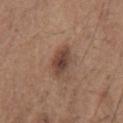Q: Was this lesion biopsied?
A: catalogued during a skin exam; not biopsied
Q: How was the tile lit?
A: white-light
Q: Where on the body is the lesion?
A: the chest
Q: Who is the patient?
A: male, in their 70s
Q: Lesion size?
A: about 4 mm
Q: What kind of image is this?
A: 15 mm crop, total-body photography